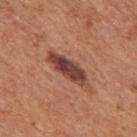This is a white-light tile.
Measured at roughly 5.5 mm in maximum diameter.
The patient is a male aged 63–67.
On the mid back.
A region of skin cropped from a whole-body photographic capture, roughly 15 mm wide.